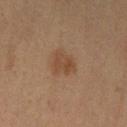<record>
  <biopsy_status>not biopsied; imaged during a skin examination</biopsy_status>
  <site>left lower leg</site>
  <patient>
    <sex>female</sex>
    <age_approx>55</age_approx>
  </patient>
  <image>
    <source>total-body photography crop</source>
    <field_of_view_mm>15</field_of_view_mm>
  </image>
  <automated_metrics>
    <eccentricity>0.6</eccentricity>
    <cielab_L>38</cielab_L>
    <cielab_a>16</cielab_a>
    <cielab_b>27</cielab_b>
    <vs_skin_darker_L>6.0</vs_skin_darker_L>
    <vs_skin_contrast_norm>6.0</vs_skin_contrast_norm>
    <border_irregularity_0_10>2.5</border_irregularity_0_10>
    <color_variation_0_10>3.0</color_variation_0_10>
    <peripheral_color_asymmetry>1.0</peripheral_color_asymmetry>
  </automated_metrics>
  <lesion_size>
    <long_diameter_mm_approx>2.5</long_diameter_mm_approx>
  </lesion_size>
  <lighting>cross-polarized</lighting>
</record>